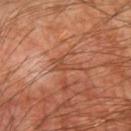The lesion was tiled from a total-body skin photograph and was not biopsied.
A roughly 15 mm field-of-view crop from a total-body skin photograph.
A male subject aged 58 to 62.
Located on the right upper arm.